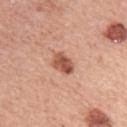{
  "automated_metrics": {
    "area_mm2_approx": 5.5,
    "shape_asymmetry": 0.2,
    "border_irregularity_0_10": 2.0,
    "color_variation_0_10": 4.5,
    "peripheral_color_asymmetry": 2.0
  },
  "lesion_size": {
    "long_diameter_mm_approx": 3.0
  },
  "patient": {
    "sex": "female",
    "age_approx": 60
  },
  "site": "left upper arm",
  "image": {
    "source": "total-body photography crop",
    "field_of_view_mm": 15
  }
}